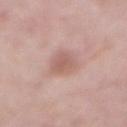Acquisition and patient details: The lesion is on the abdomen. A male subject, in their mid-50s. Automated tile analysis of the lesion measured a lesion color around L≈59 a*≈21 b*≈24 in CIELAB and about 9 CIELAB-L* units darker than the surrounding skin. The software also gave border irregularity of about 2.5 on a 0–10 scale, a color-variation rating of about 2.5/10, and peripheral color asymmetry of about 1. It also reported an automated nevus-likeness rating near 0 out of 100 and a detector confidence of about 100 out of 100 that the crop contains a lesion. Captured under white-light illumination. The lesion's longest dimension is about 3.5 mm. A region of skin cropped from a whole-body photographic capture, roughly 15 mm wide.